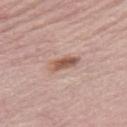Q: Is there a histopathology result?
A: imaged on a skin check; not biopsied
Q: How was this image acquired?
A: total-body-photography crop, ~15 mm field of view
Q: What is the anatomic site?
A: the right thigh
Q: What are the patient's age and sex?
A: female, aged 63 to 67